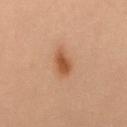Q: Was a biopsy performed?
A: imaged on a skin check; not biopsied
Q: Patient demographics?
A: female, in their 40s
Q: What kind of image is this?
A: ~15 mm crop, total-body skin-cancer survey
Q: What is the anatomic site?
A: the mid back